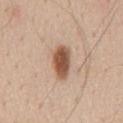Q: Is there a histopathology result?
A: no biopsy performed (imaged during a skin exam)
Q: Who is the patient?
A: male, about 60 years old
Q: What lighting was used for the tile?
A: white-light
Q: What is the anatomic site?
A: the mid back
Q: Automated lesion metrics?
A: a nevus-likeness score of about 100/100
Q: What is the lesion's diameter?
A: ~4 mm (longest diameter)
Q: What kind of image is this?
A: total-body-photography crop, ~15 mm field of view